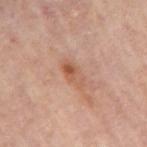The lesion was photographed on a routine skin check and not biopsied; there is no pathology result.
A 15 mm close-up tile from a total-body photography series done for melanoma screening.
Captured under cross-polarized illumination.
The recorded lesion diameter is about 3.5 mm.
The lesion is on the right upper arm.
The subject is roughly 55 years of age.